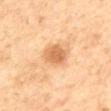Q: Is there a histopathology result?
A: imaged on a skin check; not biopsied
Q: What are the patient's age and sex?
A: female, aged around 60
Q: What is the anatomic site?
A: the upper back
Q: What is the lesion's diameter?
A: ~3.5 mm (longest diameter)
Q: What is the imaging modality?
A: 15 mm crop, total-body photography